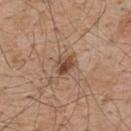Q: Was a biopsy performed?
A: catalogued during a skin exam; not biopsied
Q: What lighting was used for the tile?
A: white-light illumination
Q: What are the patient's age and sex?
A: male, roughly 55 years of age
Q: What is the anatomic site?
A: the upper back
Q: What is the imaging modality?
A: 15 mm crop, total-body photography
Q: Lesion size?
A: ≈2.5 mm